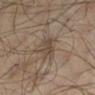A male patient, approximately 65 years of age.
Approximately 3 mm at its widest.
Cropped from a whole-body photographic skin survey; the tile spans about 15 mm.
This is a cross-polarized tile.
From the left lower leg.